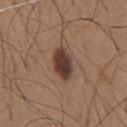workup: total-body-photography surveillance lesion; no biopsy
image source: ~15 mm crop, total-body skin-cancer survey
site: the chest
patient: male, aged around 65